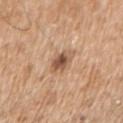Clinical summary: A male patient aged around 65. A region of skin cropped from a whole-body photographic capture, roughly 15 mm wide. Imaged with white-light lighting. The lesion is located on the right upper arm. Measured at roughly 3 mm in maximum diameter.